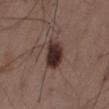Clinical impression:
Part of a total-body skin-imaging series; this lesion was reviewed on a skin check and was not flagged for biopsy.
Context:
Imaged with white-light lighting. Measured at roughly 4.5 mm in maximum diameter. A roughly 15 mm field-of-view crop from a total-body skin photograph. A male subject, aged 48 to 52. On the back.